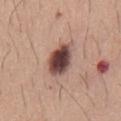- workup · imaged on a skin check; not biopsied
- location · the chest
- automated lesion analysis · a border-irregularity rating of about 2/10, a within-lesion color-variation index near 7.5/10, and peripheral color asymmetry of about 2; an automated nevus-likeness rating near 100 out of 100 and lesion-presence confidence of about 100/100
- subject · male, aged around 60
- tile lighting · white-light illumination
- size · ~4.5 mm (longest diameter)
- acquisition · total-body-photography crop, ~15 mm field of view